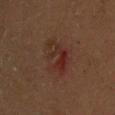workup = no biopsy performed (imaged during a skin exam); size = ≈5 mm; acquisition = ~15 mm crop, total-body skin-cancer survey; tile lighting = cross-polarized; subject = male, about 60 years old.